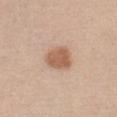Impression: Recorded during total-body skin imaging; not selected for excision or biopsy. Image and clinical context: The tile uses white-light illumination. A 15 mm close-up extracted from a 3D total-body photography capture. Longest diameter approximately 3.5 mm. Located on the chest. The patient is a female aged 23–27. The total-body-photography lesion software estimated a lesion area of about 8.5 mm², a shape eccentricity near 0.45, and a symmetry-axis asymmetry near 0.2. The software also gave a lesion color around L≈59 a*≈21 b*≈31 in CIELAB. The software also gave a border-irregularity rating of about 1.5/10, a color-variation rating of about 2.5/10, and a peripheral color-asymmetry measure near 1. It also reported a nevus-likeness score of about 100/100 and a detector confidence of about 100 out of 100 that the crop contains a lesion.